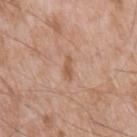notes — no biopsy performed (imaged during a skin exam)
image source — ~15 mm crop, total-body skin-cancer survey
patient — male, aged around 60
location — the mid back
size — ≈2.5 mm
tile lighting — white-light illumination